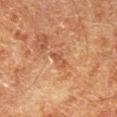This lesion was catalogued during total-body skin photography and was not selected for biopsy. Located on the leg. The patient is a male aged 58 to 62. A 15 mm close-up tile from a total-body photography series done for melanoma screening. Imaged with cross-polarized lighting. Approximately 3 mm at its widest. An algorithmic analysis of the crop reported an eccentricity of roughly 0.95 and a symmetry-axis asymmetry near 0.4. It also reported a classifier nevus-likeness of about 0/100 and lesion-presence confidence of about 95/100.